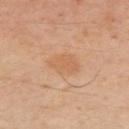- notes — catalogued during a skin exam; not biopsied
- diameter — ≈3.5 mm
- tile lighting — cross-polarized illumination
- body site — the right upper arm
- image — ~15 mm tile from a whole-body skin photo
- patient — male, in their 40s
- TBP lesion metrics — a footprint of about 8.5 mm², a shape eccentricity near 0.55, and a shape-asymmetry score of about 0.2 (0 = symmetric); roughly 6 lightness units darker than nearby skin and a lesion-to-skin contrast of about 5 (normalized; higher = more distinct); a classifier nevus-likeness of about 25/100 and a lesion-detection confidence of about 100/100